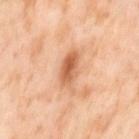Recorded during total-body skin imaging; not selected for excision or biopsy. A region of skin cropped from a whole-body photographic capture, roughly 15 mm wide. The tile uses cross-polarized illumination. Automated tile analysis of the lesion measured an area of roughly 6.5 mm² and an eccentricity of roughly 0.9. The software also gave internal color variation of about 5 on a 0–10 scale and radial color variation of about 1.5. A female subject in their mid-50s. Located on the left thigh. The lesion's longest dimension is about 4 mm.